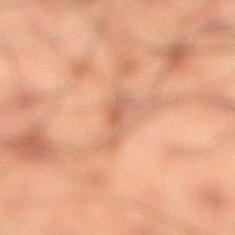The lesion was photographed on a routine skin check and not biopsied; there is no pathology result. A 15 mm crop from a total-body photograph taken for skin-cancer surveillance. Located on the right lower leg. About 4 mm across. The subject is a male aged approximately 50. An algorithmic analysis of the crop reported a lesion area of about 4.5 mm², an outline eccentricity of about 0.95 (0 = round, 1 = elongated), and two-axis asymmetry of about 0.45. The software also gave roughly 7 lightness units darker than nearby skin and a normalized border contrast of about 5.5. The analysis additionally found a border-irregularity index near 5.5/10, internal color variation of about 1.5 on a 0–10 scale, and peripheral color asymmetry of about 0. The tile uses cross-polarized illumination.